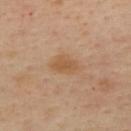The lesion-visualizer software estimated a lesion area of about 6 mm², a shape eccentricity near 0.75, and two-axis asymmetry of about 0.25. It also reported a border-irregularity index near 2.5/10 and internal color variation of about 1.5 on a 0–10 scale. The lesion is located on the upper back. A female patient, in their 40s. A close-up tile cropped from a whole-body skin photograph, about 15 mm across. The lesion's longest dimension is about 3.5 mm.